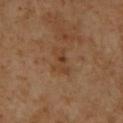Part of a total-body skin-imaging series; this lesion was reviewed on a skin check and was not flagged for biopsy. The lesion's longest dimension is about 3.5 mm. Cropped from a whole-body photographic skin survey; the tile spans about 15 mm. A female patient, approximately 50 years of age. Automated image analysis of the tile measured an area of roughly 6 mm², an eccentricity of roughly 0.85, and a shape-asymmetry score of about 0.3 (0 = symmetric). The software also gave a border-irregularity index near 3.5/10. The lesion is located on the right lower leg.